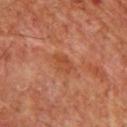Q: What is the anatomic site?
A: the chest
Q: What did automated image analysis measure?
A: an average lesion color of about L≈43 a*≈25 b*≈33 (CIELAB), about 6 CIELAB-L* units darker than the surrounding skin, and a lesion-to-skin contrast of about 6 (normalized; higher = more distinct); a color-variation rating of about 3/10 and peripheral color asymmetry of about 1; a classifier nevus-likeness of about 0/100 and a detector confidence of about 100 out of 100 that the crop contains a lesion
Q: Who is the patient?
A: male, about 60 years old
Q: What kind of image is this?
A: 15 mm crop, total-body photography
Q: Lesion size?
A: ≈3 mm
Q: How was the tile lit?
A: cross-polarized illumination